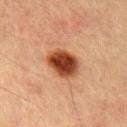biopsy_status: not biopsied; imaged during a skin examination
lesion_size:
  long_diameter_mm_approx: 4.0
image:
  source: total-body photography crop
  field_of_view_mm: 15
patient:
  sex: male
  age_approx: 55
site: left upper arm
lighting: cross-polarized